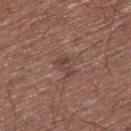Impression: The lesion was tiled from a total-body skin photograph and was not biopsied. Clinical summary: The recorded lesion diameter is about 2.5 mm. Cropped from a whole-body photographic skin survey; the tile spans about 15 mm. A male patient aged around 75. The tile uses white-light illumination. On the leg. Automated tile analysis of the lesion measured an area of roughly 3.5 mm² and a shape eccentricity near 0.8. It also reported a border-irregularity index near 4.5/10, a color-variation rating of about 2/10, and peripheral color asymmetry of about 0.5.